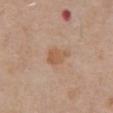Assessment:
The lesion was tiled from a total-body skin photograph and was not biopsied.
Acquisition and patient details:
A 15 mm close-up tile from a total-body photography series done for melanoma screening. A male subject aged approximately 80. This is a white-light tile. From the abdomen. The lesion's longest dimension is about 3 mm. The lesion-visualizer software estimated an area of roughly 5 mm², an outline eccentricity of about 0.65 (0 = round, 1 = elongated), and a symmetry-axis asymmetry near 0.3. The software also gave a border-irregularity index near 3/10, a color-variation rating of about 1.5/10, and peripheral color asymmetry of about 0.5. And it measured an automated nevus-likeness rating near 10 out of 100 and a lesion-detection confidence of about 100/100.